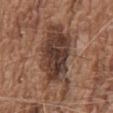No biopsy was performed on this lesion — it was imaged during a full skin examination and was not determined to be concerning.
The subject is a male aged 73 to 77.
A 15 mm close-up extracted from a 3D total-body photography capture.
On the front of the torso.